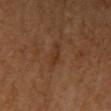biopsy status: no biopsy performed (imaged during a skin exam).